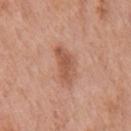The lesion was photographed on a routine skin check and not biopsied; there is no pathology result.
Automated image analysis of the tile measured a peripheral color-asymmetry measure near 1.
Located on the chest.
A male subject, in their 70s.
Approximately 4.5 mm at its widest.
A 15 mm close-up tile from a total-body photography series done for melanoma screening.
This is a white-light tile.